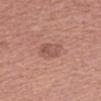Clinical impression:
This lesion was catalogued during total-body skin photography and was not selected for biopsy.
Context:
The lesion is located on the arm. A female patient, about 60 years old. A lesion tile, about 15 mm wide, cut from a 3D total-body photograph.